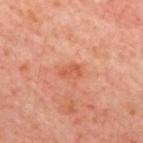Part of a total-body skin-imaging series; this lesion was reviewed on a skin check and was not flagged for biopsy.
About 2.5 mm across.
Located on the upper back.
A patient aged around 55.
A 15 mm close-up tile from a total-body photography series done for melanoma screening.
The tile uses cross-polarized illumination.